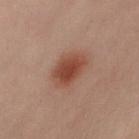Captured during whole-body skin photography for melanoma surveillance; the lesion was not biopsied.
A roughly 15 mm field-of-view crop from a total-body skin photograph.
Imaged with cross-polarized lighting.
The total-body-photography lesion software estimated a footprint of about 12 mm², a shape eccentricity near 0.6, and two-axis asymmetry of about 0.2. The software also gave a nevus-likeness score of about 100/100.
A female patient, roughly 60 years of age.
The lesion is located on the right leg.